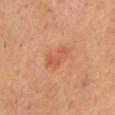Part of a total-body skin-imaging series; this lesion was reviewed on a skin check and was not flagged for biopsy. The subject is a male aged 68 to 72. A lesion tile, about 15 mm wide, cut from a 3D total-body photograph. The lesion is on the chest.